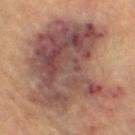The subject is a female approximately 65 years of age.
The lesion is located on the left leg.
Captured under cross-polarized illumination.
A 15 mm close-up tile from a total-body photography series done for melanoma screening.
Approximately 12 mm at its widest.